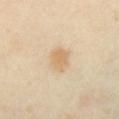Clinical impression: Part of a total-body skin-imaging series; this lesion was reviewed on a skin check and was not flagged for biopsy. Clinical summary: Measured at roughly 3 mm in maximum diameter. The subject is a female aged 38–42. A 15 mm close-up tile from a total-body photography series done for melanoma screening. Captured under cross-polarized illumination. The total-body-photography lesion software estimated a lesion color around L≈71 a*≈14 b*≈37 in CIELAB and a normalized border contrast of about 6. The analysis additionally found a lesion-detection confidence of about 100/100. On the chest.